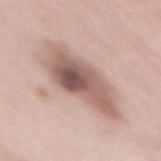| key | value |
|---|---|
| biopsy status | no biopsy performed (imaged during a skin exam) |
| patient | female, aged 63–67 |
| illumination | white-light |
| imaging modality | total-body-photography crop, ~15 mm field of view |
| anatomic site | the back |
| diameter | about 9.5 mm |